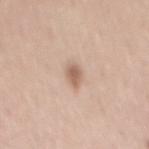Impression:
The lesion was photographed on a routine skin check and not biopsied; there is no pathology result.
Clinical summary:
The recorded lesion diameter is about 2.5 mm. The tile uses white-light illumination. The total-body-photography lesion software estimated a footprint of about 3 mm², an eccentricity of roughly 0.8, and a shape-asymmetry score of about 0.25 (0 = symmetric). It also reported border irregularity of about 2.5 on a 0–10 scale, a color-variation rating of about 1.5/10, and a peripheral color-asymmetry measure near 0.5. A male subject in their mid-40s. A roughly 15 mm field-of-view crop from a total-body skin photograph. Located on the mid back.